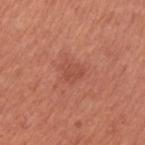workup: imaged on a skin check; not biopsied | automated metrics: a mean CIELAB color near L≈50 a*≈29 b*≈31, about 6 CIELAB-L* units darker than the surrounding skin, and a lesion-to-skin contrast of about 4.5 (normalized; higher = more distinct); a classifier nevus-likeness of about 0/100 and lesion-presence confidence of about 100/100 | diameter: ~2.5 mm (longest diameter) | image: ~15 mm tile from a whole-body skin photo | patient: female, in their mid-60s | tile lighting: white-light | anatomic site: the left upper arm.